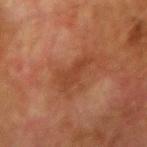Part of a total-body skin-imaging series; this lesion was reviewed on a skin check and was not flagged for biopsy. Longest diameter approximately 4.5 mm. The lesion is located on the right upper arm. Cropped from a whole-body photographic skin survey; the tile spans about 15 mm. This is a cross-polarized tile. The lesion-visualizer software estimated an average lesion color of about L≈35 a*≈22 b*≈29 (CIELAB), roughly 6 lightness units darker than nearby skin, and a normalized lesion–skin contrast near 6. The software also gave a border-irregularity rating of about 6/10 and a within-lesion color-variation index near 1/10. The software also gave a classifier nevus-likeness of about 0/100 and a lesion-detection confidence of about 100/100. The subject is a male roughly 75 years of age.